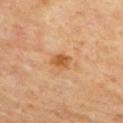<tbp_lesion>
<biopsy_status>not biopsied; imaged during a skin examination</biopsy_status>
<lesion_size>
  <long_diameter_mm_approx>2.5</long_diameter_mm_approx>
</lesion_size>
<site>upper back</site>
<patient>
  <sex>female</sex>
  <age_approx>65</age_approx>
</patient>
<lighting>cross-polarized</lighting>
<image>
  <source>total-body photography crop</source>
  <field_of_view_mm>15</field_of_view_mm>
</image>
</tbp_lesion>